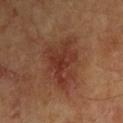biopsy status = no biopsy performed (imaged during a skin exam).